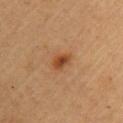Imaged during a routine full-body skin examination; the lesion was not biopsied and no histopathology is available.
A close-up tile cropped from a whole-body skin photograph, about 15 mm across.
The tile uses cross-polarized illumination.
The lesion is on the left upper arm.
An algorithmic analysis of the crop reported a lesion area of about 3.5 mm², an eccentricity of roughly 0.75, and a shape-asymmetry score of about 0.3 (0 = symmetric). And it measured a normalized border contrast of about 9. The software also gave a border-irregularity index near 2.5/10, a within-lesion color-variation index near 3/10, and peripheral color asymmetry of about 1. The software also gave an automated nevus-likeness rating near 95 out of 100.
A female subject aged 58 to 62.
The recorded lesion diameter is about 2.5 mm.